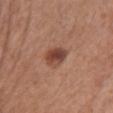Captured during whole-body skin photography for melanoma surveillance; the lesion was not biopsied. This is a white-light tile. A lesion tile, about 15 mm wide, cut from a 3D total-body photograph. Automated tile analysis of the lesion measured a footprint of about 6 mm² and two-axis asymmetry of about 0.15. The analysis additionally found an average lesion color of about L≈44 a*≈23 b*≈28 (CIELAB), about 12 CIELAB-L* units darker than the surrounding skin, and a normalized border contrast of about 9. And it measured a border-irregularity rating of about 1.5/10, a color-variation rating of about 6/10, and radial color variation of about 2. The software also gave a classifier nevus-likeness of about 95/100 and lesion-presence confidence of about 100/100. A female subject, aged 68 to 72. Longest diameter approximately 3 mm. The lesion is located on the chest.